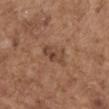Impression:
This lesion was catalogued during total-body skin photography and was not selected for biopsy.
Image and clinical context:
About 4 mm across. A female subject, about 65 years old. Automated tile analysis of the lesion measured a footprint of about 6.5 mm², an eccentricity of roughly 0.9, and two-axis asymmetry of about 0.25. And it measured a border-irregularity index near 3/10 and a color-variation rating of about 5/10. It also reported a lesion-detection confidence of about 100/100. On the mid back. A roughly 15 mm field-of-view crop from a total-body skin photograph.